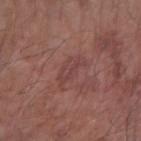biopsy status — no biopsy performed (imaged during a skin exam) | image — ~15 mm crop, total-body skin-cancer survey | lighting — white-light | patient — male, in their 80s | site — the right forearm.